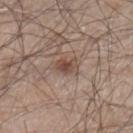Q: Is there a histopathology result?
A: imaged on a skin check; not biopsied
Q: What did automated image analysis measure?
A: a lesion color around L≈47 a*≈17 b*≈25 in CIELAB, roughly 10 lightness units darker than nearby skin, and a normalized lesion–skin contrast near 7.5; an automated nevus-likeness rating near 85 out of 100 and a lesion-detection confidence of about 100/100
Q: Patient demographics?
A: male, roughly 45 years of age
Q: What is the lesion's diameter?
A: about 2.5 mm
Q: Lesion location?
A: the right lower leg
Q: How was this image acquired?
A: ~15 mm tile from a whole-body skin photo